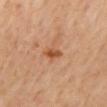Imaged with cross-polarized lighting.
The lesion is on the back.
Measured at roughly 2.5 mm in maximum diameter.
This image is a 15 mm lesion crop taken from a total-body photograph.
A male patient in their mid-60s.